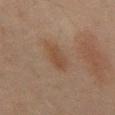Impression: No biopsy was performed on this lesion — it was imaged during a full skin examination and was not determined to be concerning. Image and clinical context: Cropped from a whole-body photographic skin survey; the tile spans about 15 mm. An algorithmic analysis of the crop reported a mean CIELAB color near L≈37 a*≈14 b*≈25 and a normalized lesion–skin contrast near 5.5. And it measured a border-irregularity rating of about 2.5/10, a within-lesion color-variation index near 2.5/10, and radial color variation of about 1. It also reported an automated nevus-likeness rating near 5 out of 100 and a detector confidence of about 100 out of 100 that the crop contains a lesion. The tile uses cross-polarized illumination. On the mid back. Longest diameter approximately 4 mm. A male patient, approximately 45 years of age.